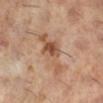| field | value |
|---|---|
| biopsy status | total-body-photography surveillance lesion; no biopsy |
| anatomic site | the right lower leg |
| patient | female, approximately 60 years of age |
| acquisition | ~15 mm crop, total-body skin-cancer survey |